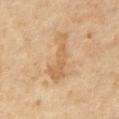biopsy status: total-body-photography surveillance lesion; no biopsy
patient: male, about 65 years old
image: ~15 mm tile from a whole-body skin photo
tile lighting: cross-polarized illumination
TBP lesion metrics: a lesion area of about 12 mm² and a shape-asymmetry score of about 0.5 (0 = symmetric); roughly 8 lightness units darker than nearby skin and a lesion-to-skin contrast of about 5.5 (normalized; higher = more distinct); a color-variation rating of about 2.5/10
diameter: ≈6.5 mm